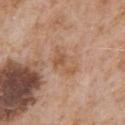Q: Is there a histopathology result?
A: total-body-photography surveillance lesion; no biopsy
Q: How was the tile lit?
A: white-light illumination
Q: Where on the body is the lesion?
A: the chest
Q: How large is the lesion?
A: about 4 mm
Q: What kind of image is this?
A: ~15 mm crop, total-body skin-cancer survey
Q: Who is the patient?
A: male, in their mid- to late 60s